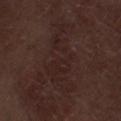| feature | finding |
|---|---|
| diameter | ≈9 mm |
| image-analysis metrics | an outline eccentricity of about 0.95 (0 = round, 1 = elongated) and two-axis asymmetry of about 0.5; a mean CIELAB color near L≈22 a*≈16 b*≈17, about 4 CIELAB-L* units darker than the surrounding skin, and a lesion-to-skin contrast of about 5 (normalized; higher = more distinct); a border-irregularity rating of about 8.5/10 and a within-lesion color-variation index near 2.5/10; a nevus-likeness score of about 0/100 |
| image source | 15 mm crop, total-body photography |
| location | the right thigh |
| subject | male, roughly 70 years of age |